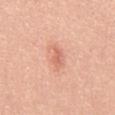Assessment:
Part of a total-body skin-imaging series; this lesion was reviewed on a skin check and was not flagged for biopsy.
Image and clinical context:
The lesion's longest dimension is about 3.5 mm. Captured under white-light illumination. On the front of the torso. Automated tile analysis of the lesion measured an eccentricity of roughly 0.9 and a shape-asymmetry score of about 0.3 (0 = symmetric). And it measured an average lesion color of about L≈66 a*≈27 b*≈32 (CIELAB) and roughly 9 lightness units darker than nearby skin. A 15 mm crop from a total-body photograph taken for skin-cancer surveillance. A male subject in their mid- to late 40s.